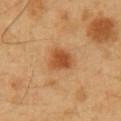Captured during whole-body skin photography for melanoma surveillance; the lesion was not biopsied.